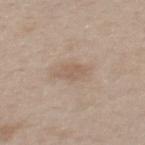Impression: This lesion was catalogued during total-body skin photography and was not selected for biopsy. Clinical summary: A close-up tile cropped from a whole-body skin photograph, about 15 mm across. Measured at roughly 2.5 mm in maximum diameter. The patient is a female aged 38–42. An algorithmic analysis of the crop reported a lesion area of about 4 mm², an outline eccentricity of about 0.7 (0 = round, 1 = elongated), and two-axis asymmetry of about 0.25. It also reported a color-variation rating of about 1.5/10. It also reported an automated nevus-likeness rating near 15 out of 100. The lesion is located on the mid back.